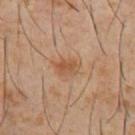subject = male, in their 60s | illumination = cross-polarized illumination | imaging modality = total-body-photography crop, ~15 mm field of view | diameter = ~2.5 mm (longest diameter) | automated lesion analysis = a lesion color around L≈49 a*≈20 b*≈32 in CIELAB, about 8 CIELAB-L* units darker than the surrounding skin, and a normalized border contrast of about 6.5 | location = the abdomen.